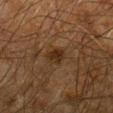Q: Was a biopsy performed?
A: imaged on a skin check; not biopsied
Q: Automated lesion metrics?
A: a lesion–skin lightness drop of about 7 and a lesion-to-skin contrast of about 8.5 (normalized; higher = more distinct)
Q: What are the patient's age and sex?
A: male, roughly 65 years of age
Q: What is the anatomic site?
A: the arm
Q: How was this image acquired?
A: ~15 mm crop, total-body skin-cancer survey
Q: How large is the lesion?
A: ~2.5 mm (longest diameter)